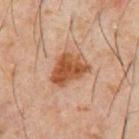<record>
<biopsy_status>not biopsied; imaged during a skin examination</biopsy_status>
<patient>
  <sex>male</sex>
  <age_approx>60</age_approx>
</patient>
<image>
  <source>total-body photography crop</source>
  <field_of_view_mm>15</field_of_view_mm>
</image>
<lesion_size>
  <long_diameter_mm_approx>4.5</long_diameter_mm_approx>
</lesion_size>
<site>abdomen</site>
<automated_metrics>
  <border_irregularity_0_10>3.0</border_irregularity_0_10>
  <color_variation_0_10>4.5</color_variation_0_10>
  <peripheral_color_asymmetry>1.5</peripheral_color_asymmetry>
  <nevus_likeness_0_100>95</nevus_likeness_0_100>
</automated_metrics>
<lighting>cross-polarized</lighting>
</record>